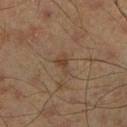Part of a total-body skin-imaging series; this lesion was reviewed on a skin check and was not flagged for biopsy. A male subject aged around 45. A region of skin cropped from a whole-body photographic capture, roughly 15 mm wide. The lesion's longest dimension is about 2.5 mm. Located on the right lower leg.